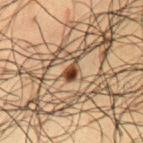This lesion was catalogued during total-body skin photography and was not selected for biopsy. A male subject, aged 58 to 62. The lesion-visualizer software estimated a lesion color around L≈32 a*≈17 b*≈26 in CIELAB, a lesion–skin lightness drop of about 14, and a normalized lesion–skin contrast near 12.5. A 15 mm close-up tile from a total-body photography series done for melanoma screening. Approximately 2.5 mm at its widest. The lesion is located on the front of the torso.